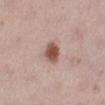* workup — total-body-photography surveillance lesion; no biopsy
* site — the leg
* image source — 15 mm crop, total-body photography
* size — ~3 mm (longest diameter)
* patient — female, aged 38 to 42